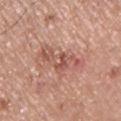{
  "biopsy_status": "not biopsied; imaged during a skin examination",
  "lighting": "white-light",
  "automated_metrics": {
    "area_mm2_approx": 12.0,
    "eccentricity": 0.95,
    "shape_asymmetry": 0.4,
    "cielab_L": 55,
    "cielab_a": 24,
    "cielab_b": 27,
    "vs_skin_darker_L": 10.0,
    "vs_skin_contrast_norm": 6.5,
    "nevus_likeness_0_100": 0,
    "lesion_detection_confidence_0_100": 100
  },
  "site": "right upper arm",
  "patient": {
    "sex": "male",
    "age_approx": 55
  },
  "image": {
    "source": "total-body photography crop",
    "field_of_view_mm": 15
  }
}